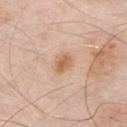Impression:
The lesion was photographed on a routine skin check and not biopsied; there is no pathology result.
Context:
A male patient aged 53 to 57. Located on the chest. A close-up tile cropped from a whole-body skin photograph, about 15 mm across. An algorithmic analysis of the crop reported an area of roughly 5.5 mm² and a symmetry-axis asymmetry near 0.2. It also reported a nevus-likeness score of about 50/100. The tile uses white-light illumination.